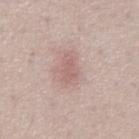Measured at roughly 3.5 mm in maximum diameter. A close-up tile cropped from a whole-body skin photograph, about 15 mm across. Located on the abdomen. The total-body-photography lesion software estimated a nevus-likeness score of about 5/100 and lesion-presence confidence of about 100/100. The tile uses white-light illumination. A male patient, aged around 50.